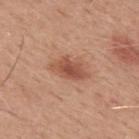acquisition: total-body-photography crop, ~15 mm field of view
lesion size: ≈4.5 mm
automated metrics: an average lesion color of about L≈52 a*≈25 b*≈31 (CIELAB), a lesion–skin lightness drop of about 11, and a normalized lesion–skin contrast near 7.5; a border-irregularity index near 2.5/10, a within-lesion color-variation index near 4.5/10, and a peripheral color-asymmetry measure near 1.5; a nevus-likeness score of about 80/100 and lesion-presence confidence of about 100/100
anatomic site: the back
subject: male, aged 48–52